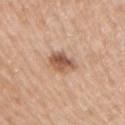biopsy status: catalogued during a skin exam; not biopsied | body site: the upper back | imaging modality: ~15 mm crop, total-body skin-cancer survey | size: ~3.5 mm (longest diameter) | lighting: white-light | TBP lesion metrics: border irregularity of about 2.5 on a 0–10 scale, internal color variation of about 5.5 on a 0–10 scale, and radial color variation of about 2 | patient: male, roughly 50 years of age.